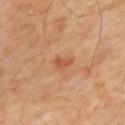The lesion was tiled from a total-body skin photograph and was not biopsied.
A male patient aged 53–57.
The lesion-visualizer software estimated a footprint of about 3 mm², a shape eccentricity near 0.8, and two-axis asymmetry of about 0.35. The analysis additionally found border irregularity of about 3 on a 0–10 scale, internal color variation of about 1.5 on a 0–10 scale, and a peripheral color-asymmetry measure near 0.5.
The recorded lesion diameter is about 2.5 mm.
Imaged with cross-polarized lighting.
Cropped from a total-body skin-imaging series; the visible field is about 15 mm.
Located on the mid back.